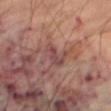{"biopsy_status": "not biopsied; imaged during a skin examination", "site": "leg", "image": {"source": "total-body photography crop", "field_of_view_mm": 15}, "patient": {"sex": "male", "age_approx": 70}, "lighting": "cross-polarized", "automated_metrics": {"area_mm2_approx": 6.0, "eccentricity": 0.75, "shape_asymmetry": 0.45, "border_irregularity_0_10": 5.0, "peripheral_color_asymmetry": 2.0, "nevus_likeness_0_100": 0, "lesion_detection_confidence_0_100": 65}}